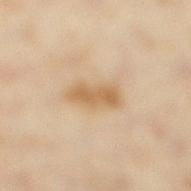{
  "biopsy_status": "not biopsied; imaged during a skin examination",
  "lesion_size": {
    "long_diameter_mm_approx": 4.5
  },
  "lighting": "cross-polarized",
  "site": "right lower leg",
  "automated_metrics": {
    "area_mm2_approx": 7.5,
    "eccentricity": 0.9,
    "vs_skin_darker_L": 10.0,
    "vs_skin_contrast_norm": 7.5,
    "border_irregularity_0_10": 2.5,
    "peripheral_color_asymmetry": 0.5,
    "nevus_likeness_0_100": 10,
    "lesion_detection_confidence_0_100": 100
  },
  "patient": {
    "sex": "female",
    "age_approx": 35
  },
  "image": {
    "source": "total-body photography crop",
    "field_of_view_mm": 15
  }
}